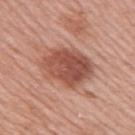follow-up: no biopsy performed (imaged during a skin exam) | body site: the right upper arm | imaging modality: ~15 mm tile from a whole-body skin photo | tile lighting: white-light illumination | diameter: about 5.5 mm | subject: female, aged 38–42 | automated metrics: an area of roughly 19 mm² and a shape-asymmetry score of about 0.2 (0 = symmetric); roughly 13 lightness units darker than nearby skin; an automated nevus-likeness rating near 80 out of 100 and lesion-presence confidence of about 100/100.